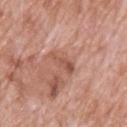Impression:
Captured during whole-body skin photography for melanoma surveillance; the lesion was not biopsied.
Clinical summary:
Automated tile analysis of the lesion measured a lesion area of about 3.5 mm², a shape eccentricity near 0.9, and a symmetry-axis asymmetry near 0.4. It also reported a nevus-likeness score of about 0/100 and a lesion-detection confidence of about 100/100. From the upper back. The tile uses white-light illumination. The recorded lesion diameter is about 3.5 mm. A male subject approximately 70 years of age. A close-up tile cropped from a whole-body skin photograph, about 15 mm across.